A close-up tile cropped from a whole-body skin photograph, about 15 mm across. The lesion is located on the left upper arm. A male patient roughly 70 years of age:
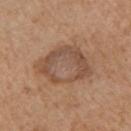Diagnosis:
Biopsy histopathology demonstrated a seborrheic keratosis.